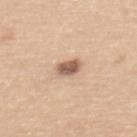Q: Was a biopsy performed?
A: catalogued during a skin exam; not biopsied
Q: What are the patient's age and sex?
A: female, about 65 years old
Q: What is the imaging modality?
A: total-body-photography crop, ~15 mm field of view
Q: How was the tile lit?
A: white-light illumination
Q: What is the anatomic site?
A: the mid back